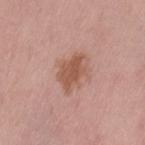Case summary:
* follow-up — no biopsy performed (imaged during a skin exam)
* patient — female, in their mid- to late 60s
* imaging modality — ~15 mm tile from a whole-body skin photo
* body site — the left thigh